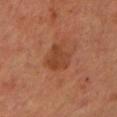Clinical impression: Imaged during a routine full-body skin examination; the lesion was not biopsied and no histopathology is available. Background: The tile uses cross-polarized illumination. Approximately 3.5 mm at its widest. On the upper back. The patient is a male aged 58 to 62. This image is a 15 mm lesion crop taken from a total-body photograph.